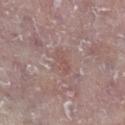biopsy status: total-body-photography surveillance lesion; no biopsy | image source: ~15 mm crop, total-body skin-cancer survey | body site: the right lower leg | subject: male, aged around 75 | automated lesion analysis: a lesion area of about 3.5 mm², an outline eccentricity of about 0.85 (0 = round, 1 = elongated), and a symmetry-axis asymmetry near 0.25; a border-irregularity index near 3/10, internal color variation of about 1.5 on a 0–10 scale, and peripheral color asymmetry of about 0 | size: about 2.5 mm.